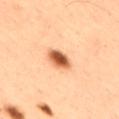Impression:
Part of a total-body skin-imaging series; this lesion was reviewed on a skin check and was not flagged for biopsy.
Clinical summary:
A close-up tile cropped from a whole-body skin photograph, about 15 mm across. About 3 mm across. Automated image analysis of the tile measured a shape eccentricity near 0.65 and a symmetry-axis asymmetry near 0.2. The analysis additionally found about 20 CIELAB-L* units darker than the surrounding skin and a normalized border contrast of about 11.5. A male patient aged 48 to 52. Located on the right thigh.